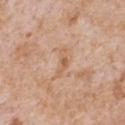  biopsy_status: not biopsied; imaged during a skin examination
  patient:
    sex: male
    age_approx: 65
  site: chest
  lesion_size:
    long_diameter_mm_approx: 3.5
  lighting: white-light
  image:
    source: total-body photography crop
    field_of_view_mm: 15